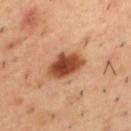Clinical impression:
This lesion was catalogued during total-body skin photography and was not selected for biopsy.
Background:
A region of skin cropped from a whole-body photographic capture, roughly 15 mm wide. From the back. A male patient aged 53 to 57. Automated tile analysis of the lesion measured a lesion area of about 11 mm², a shape eccentricity near 0.8, and two-axis asymmetry of about 0.2. The software also gave an average lesion color of about L≈47 a*≈26 b*≈35 (CIELAB), about 17 CIELAB-L* units darker than the surrounding skin, and a normalized border contrast of about 12. And it measured lesion-presence confidence of about 100/100.